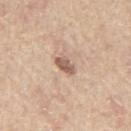Clinical impression:
This lesion was catalogued during total-body skin photography and was not selected for biopsy.
Acquisition and patient details:
The lesion's longest dimension is about 3 mm. A male subject, aged approximately 65. Captured under white-light illumination. A 15 mm crop from a total-body photograph taken for skin-cancer surveillance. An algorithmic analysis of the crop reported an area of roughly 4 mm², an eccentricity of roughly 0.8, and two-axis asymmetry of about 0.3. The software also gave about 13 CIELAB-L* units darker than the surrounding skin and a normalized lesion–skin contrast near 8.5. It also reported border irregularity of about 3 on a 0–10 scale and peripheral color asymmetry of about 1. On the mid back.